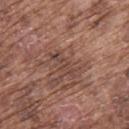Captured during whole-body skin photography for melanoma surveillance; the lesion was not biopsied. A 15 mm crop from a total-body photograph taken for skin-cancer surveillance. A male subject, about 75 years old. From the upper back.